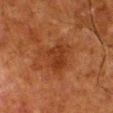The lesion was photographed on a routine skin check and not biopsied; there is no pathology result. A male subject approximately 80 years of age. The lesion is on the right lower leg. A 15 mm close-up tile from a total-body photography series done for melanoma screening.